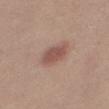This lesion was catalogued during total-body skin photography and was not selected for biopsy.
Automated image analysis of the tile measured a mean CIELAB color near L≈52 a*≈21 b*≈25, a lesion–skin lightness drop of about 10, and a lesion-to-skin contrast of about 7.5 (normalized; higher = more distinct). The software also gave border irregularity of about 2 on a 0–10 scale and internal color variation of about 2 on a 0–10 scale.
A close-up tile cropped from a whole-body skin photograph, about 15 mm across.
A female patient, aged 18–22.
The tile uses white-light illumination.
Approximately 4 mm at its widest.
The lesion is on the right thigh.